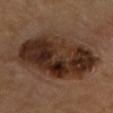This lesion was catalogued during total-body skin photography and was not selected for biopsy. This is a cross-polarized tile. A 15 mm crop from a total-body photograph taken for skin-cancer surveillance. On the chest. Automated image analysis of the tile measured a mean CIELAB color near L≈30 a*≈18 b*≈25, roughly 13 lightness units darker than nearby skin, and a normalized border contrast of about 12.5. It also reported a border-irregularity rating of about 2.5/10 and internal color variation of about 8.5 on a 0–10 scale.